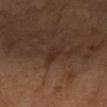Findings:
* workup — catalogued during a skin exam; not biopsied
* acquisition — ~15 mm tile from a whole-body skin photo
* subject — female, aged around 60
* tile lighting — cross-polarized
* anatomic site — the left forearm
* lesion size — ≈2.5 mm